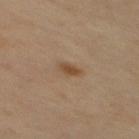  biopsy_status: not biopsied; imaged during a skin examination